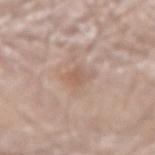  patient:
    sex: male
    age_approx: 80
  lesion_size:
    long_diameter_mm_approx: 3.0
  image:
    source: total-body photography crop
    field_of_view_mm: 15
  site: left arm